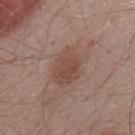• biopsy status — imaged on a skin check; not biopsied
• subject — male, in their mid-50s
• lesion size — ~4.5 mm (longest diameter)
• TBP lesion metrics — a lesion color around L≈47 a*≈19 b*≈25 in CIELAB, about 8 CIELAB-L* units darker than the surrounding skin, and a normalized border contrast of about 6.5; a border-irregularity index near 2/10, a color-variation rating of about 3.5/10, and radial color variation of about 1; an automated nevus-likeness rating near 30 out of 100 and a lesion-detection confidence of about 100/100
• location — the mid back
• image source — total-body-photography crop, ~15 mm field of view